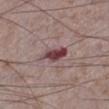Recorded during total-body skin imaging; not selected for excision or biopsy.
A close-up tile cropped from a whole-body skin photograph, about 15 mm across.
Automated tile analysis of the lesion measured a footprint of about 6 mm², a shape eccentricity near 0.9, and a shape-asymmetry score of about 0.35 (0 = symmetric).
A male subject, aged 73 to 77.
The tile uses white-light illumination.
From the left lower leg.